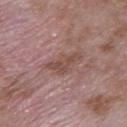Image and clinical context:
This image is a 15 mm lesion crop taken from a total-body photograph. Captured under white-light illumination. The lesion's longest dimension is about 4 mm. Located on the upper back. A male subject aged around 50.